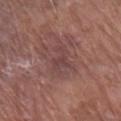biopsy status = no biopsy performed (imaged during a skin exam)
anatomic site = the left forearm
image source = ~15 mm crop, total-body skin-cancer survey
size = ≈5 mm
patient = male, aged approximately 80